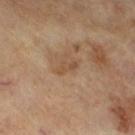The lesion-visualizer software estimated a border-irregularity index near 5/10 and a peripheral color-asymmetry measure near 0. The analysis additionally found a nevus-likeness score of about 0/100.
The patient is a male in their mid- to late 60s.
Cropped from a whole-body photographic skin survey; the tile spans about 15 mm.
About 2.5 mm across.